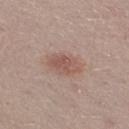Q: Is there a histopathology result?
A: total-body-photography surveillance lesion; no biopsy